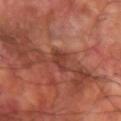A male subject, about 60 years old. From the left forearm. The lesion's longest dimension is about 3 mm. Imaged with cross-polarized lighting. Cropped from a total-body skin-imaging series; the visible field is about 15 mm. Automated tile analysis of the lesion measured a lesion area of about 4 mm², an outline eccentricity of about 0.75 (0 = round, 1 = elongated), and a shape-asymmetry score of about 0.35 (0 = symmetric). The analysis additionally found a lesion color around L≈38 a*≈27 b*≈28 in CIELAB, a lesion–skin lightness drop of about 8, and a lesion-to-skin contrast of about 6.5 (normalized; higher = more distinct). The software also gave internal color variation of about 1.5 on a 0–10 scale and peripheral color asymmetry of about 0.5. The analysis additionally found an automated nevus-likeness rating near 0 out of 100.